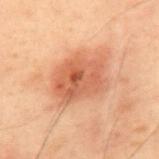Case summary:
• workup: total-body-photography surveillance lesion; no biopsy
• lighting: cross-polarized illumination
• TBP lesion metrics: a border-irregularity rating of about 2/10, a color-variation rating of about 5.5/10, and a peripheral color-asymmetry measure near 2
• image source: total-body-photography crop, ~15 mm field of view
• subject: male, aged around 55
• location: the mid back
• size: about 5.5 mm